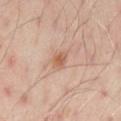Q: Was this lesion biopsied?
A: no biopsy performed (imaged during a skin exam)
Q: Who is the patient?
A: male, roughly 50 years of age
Q: What is the imaging modality?
A: ~15 mm tile from a whole-body skin photo
Q: How large is the lesion?
A: ~2.5 mm (longest diameter)
Q: How was the tile lit?
A: cross-polarized
Q: What is the anatomic site?
A: the front of the torso